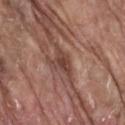Recorded during total-body skin imaging; not selected for excision or biopsy. This is a white-light tile. The lesion is located on the lower back. The patient is a male approximately 80 years of age. Longest diameter approximately 2.5 mm. This image is a 15 mm lesion crop taken from a total-body photograph.